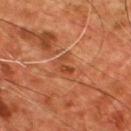Assessment:
This lesion was catalogued during total-body skin photography and was not selected for biopsy.
Context:
This is a cross-polarized tile. Located on the front of the torso. Longest diameter approximately 2.5 mm. A male subject, approximately 55 years of age. A 15 mm close-up tile from a total-body photography series done for melanoma screening.